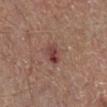This lesion was catalogued during total-body skin photography and was not selected for biopsy. The tile uses white-light illumination. From the right lower leg. The lesion's longest dimension is about 2.5 mm. The patient is a male aged around 55. A lesion tile, about 15 mm wide, cut from a 3D total-body photograph.